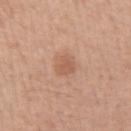Part of a total-body skin-imaging series; this lesion was reviewed on a skin check and was not flagged for biopsy. A region of skin cropped from a whole-body photographic capture, roughly 15 mm wide. A male patient roughly 45 years of age. Imaged with white-light lighting. The lesion is located on the left upper arm. Measured at roughly 2.5 mm in maximum diameter.